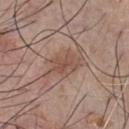Imaged during a routine full-body skin examination; the lesion was not biopsied and no histopathology is available.
Imaged with white-light lighting.
A male subject aged approximately 55.
A 15 mm close-up tile from a total-body photography series done for melanoma screening.
The total-body-photography lesion software estimated a border-irregularity rating of about 5/10 and peripheral color asymmetry of about 1.
From the chest.